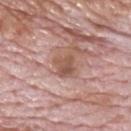Findings:
* subject: male, aged approximately 70
* imaging modality: 15 mm crop, total-body photography
* site: the upper back